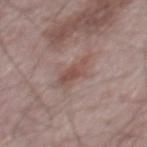This lesion was catalogued during total-body skin photography and was not selected for biopsy. The patient is a male roughly 65 years of age. Located on the mid back. About 4.5 mm across. The total-body-photography lesion software estimated a lesion-detection confidence of about 100/100. A close-up tile cropped from a whole-body skin photograph, about 15 mm across.